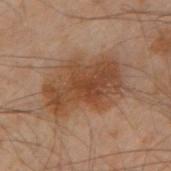The lesion was tiled from a total-body skin photograph and was not biopsied.
Automated tile analysis of the lesion measured a border-irregularity index near 3.5/10, internal color variation of about 4.5 on a 0–10 scale, and a peripheral color-asymmetry measure near 1.5.
A male patient aged 48 to 52.
The tile uses cross-polarized illumination.
A 15 mm close-up extracted from a 3D total-body photography capture.
The recorded lesion diameter is about 8 mm.
The lesion is on the arm.